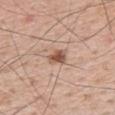| field | value |
|---|---|
| workup | imaged on a skin check; not biopsied |
| automated lesion analysis | a shape eccentricity near 0.7; a nevus-likeness score of about 95/100 and lesion-presence confidence of about 100/100 |
| illumination | white-light |
| subject | male, in their mid-60s |
| imaging modality | total-body-photography crop, ~15 mm field of view |
| size | ~2.5 mm (longest diameter) |
| site | the mid back |